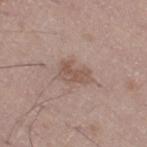follow-up — imaged on a skin check; not biopsied | imaging modality — total-body-photography crop, ~15 mm field of view | subject — male, in their 50s | lesion diameter — about 4 mm | body site — the right thigh.